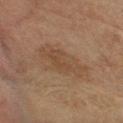Assessment:
Part of a total-body skin-imaging series; this lesion was reviewed on a skin check and was not flagged for biopsy.
Clinical summary:
A lesion tile, about 15 mm wide, cut from a 3D total-body photograph. A female patient about 60 years old. Located on the head or neck. Imaged with cross-polarized lighting.